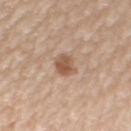Part of a total-body skin-imaging series; this lesion was reviewed on a skin check and was not flagged for biopsy. This is a white-light tile. A roughly 15 mm field-of-view crop from a total-body skin photograph. On the arm. A female subject aged 48 to 52. An algorithmic analysis of the crop reported an eccentricity of roughly 0.4 and two-axis asymmetry of about 0.2. And it measured an automated nevus-likeness rating near 75 out of 100. The lesion's longest dimension is about 2.5 mm.